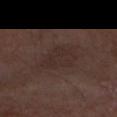Q: Was this lesion biopsied?
A: imaged on a skin check; not biopsied
Q: Who is the patient?
A: male, approximately 75 years of age
Q: Automated lesion metrics?
A: a lesion area of about 13 mm²; roughly 4 lightness units darker than nearby skin and a lesion-to-skin contrast of about 5 (normalized; higher = more distinct)
Q: Where on the body is the lesion?
A: the right forearm
Q: How large is the lesion?
A: ≈5 mm
Q: What is the imaging modality?
A: 15 mm crop, total-body photography